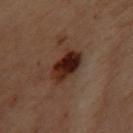Notes:
– notes · catalogued during a skin exam; not biopsied
– image · ~15 mm tile from a whole-body skin photo
– subject · female, aged around 60
– location · the upper back